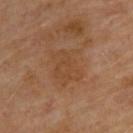Clinical impression:
No biopsy was performed on this lesion — it was imaged during a full skin examination and was not determined to be concerning.
Clinical summary:
A male subject, aged 68 to 72. Captured under cross-polarized illumination. A 15 mm close-up extracted from a 3D total-body photography capture. Located on the upper back.